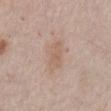| key | value |
|---|---|
| workup | imaged on a skin check; not biopsied |
| image-analysis metrics | an area of roughly 6.5 mm² and a shape eccentricity near 0.85 |
| patient | male, in their mid-60s |
| image source | total-body-photography crop, ~15 mm field of view |
| site | the abdomen |